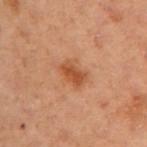Recorded during total-body skin imaging; not selected for excision or biopsy. About 3.5 mm across. Automated image analysis of the tile measured a symmetry-axis asymmetry near 0.25. The software also gave a lesion color around L≈43 a*≈23 b*≈32 in CIELAB, roughly 9 lightness units darker than nearby skin, and a lesion-to-skin contrast of about 7.5 (normalized; higher = more distinct). And it measured a classifier nevus-likeness of about 35/100 and a detector confidence of about 100 out of 100 that the crop contains a lesion. On the left upper arm. The subject is a female approximately 55 years of age. A close-up tile cropped from a whole-body skin photograph, about 15 mm across.